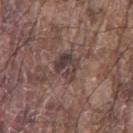biopsy status: catalogued during a skin exam; not biopsied | body site: the left thigh | illumination: white-light illumination | acquisition: 15 mm crop, total-body photography | automated lesion analysis: a lesion area of about 7.5 mm², an outline eccentricity of about 0.6 (0 = round, 1 = elongated), and two-axis asymmetry of about 0.25; an average lesion color of about L≈39 a*≈15 b*≈18 (CIELAB) and roughly 9 lightness units darker than nearby skin; a border-irregularity rating of about 2.5/10 and internal color variation of about 7 on a 0–10 scale; a nevus-likeness score of about 0/100 and a lesion-detection confidence of about 60/100 | subject: male, aged approximately 80.